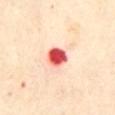<lesion>
  <biopsy_status>not biopsied; imaged during a skin examination</biopsy_status>
  <image>
    <source>total-body photography crop</source>
    <field_of_view_mm>15</field_of_view_mm>
  </image>
  <patient>
    <sex>female</sex>
    <age_approx>60</age_approx>
  </patient>
  <lighting>cross-polarized</lighting>
  <site>abdomen</site>
  <automated_metrics>
    <area_mm2_approx>6.0</area_mm2_approx>
    <eccentricity>0.4</eccentricity>
    <shape_asymmetry>0.15</shape_asymmetry>
    <nevus_likeness_0_100>0</nevus_likeness_0_100>
  </automated_metrics>
  <lesion_size>
    <long_diameter_mm_approx>3.0</long_diameter_mm_approx>
  </lesion_size>
</lesion>